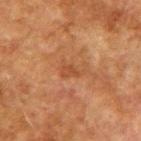{"biopsy_status": "not biopsied; imaged during a skin examination", "patient": {"sex": "male", "age_approx": 75}, "image": {"source": "total-body photography crop", "field_of_view_mm": 15}, "site": "right upper arm"}